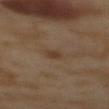Q: Was a biopsy performed?
A: imaged on a skin check; not biopsied
Q: What kind of image is this?
A: 15 mm crop, total-body photography
Q: What lighting was used for the tile?
A: cross-polarized
Q: Who is the patient?
A: female, aged 53 to 57
Q: What did automated image analysis measure?
A: a footprint of about 5 mm²; a border-irregularity index near 2.5/10, internal color variation of about 2 on a 0–10 scale, and a peripheral color-asymmetry measure near 0.5
Q: What is the anatomic site?
A: the mid back
Q: Lesion size?
A: ≈2.5 mm